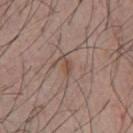Impression: Captured during whole-body skin photography for melanoma surveillance; the lesion was not biopsied. Clinical summary: On the front of the torso. A region of skin cropped from a whole-body photographic capture, roughly 15 mm wide. The subject is a male in their mid- to late 50s. This is a white-light tile. An algorithmic analysis of the crop reported a footprint of about 2.5 mm² and a shape-asymmetry score of about 0.35 (0 = symmetric). The analysis additionally found a lesion-detection confidence of about 100/100.